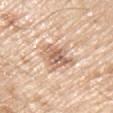biopsy status — imaged on a skin check; not biopsied
location — the right upper arm
image-analysis metrics — an outline eccentricity of about 0.45 (0 = round, 1 = elongated) and two-axis asymmetry of about 0.35; a lesion color around L≈65 a*≈19 b*≈31 in CIELAB, roughly 13 lightness units darker than nearby skin, and a normalized lesion–skin contrast near 7.5
subject — male, aged around 80
lighting — white-light illumination
imaging modality — total-body-photography crop, ~15 mm field of view
diameter — ~3.5 mm (longest diameter)